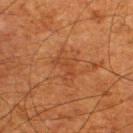Case summary:
* workup · total-body-photography surveillance lesion; no biopsy
* automated metrics · an area of roughly 5.5 mm², an outline eccentricity of about 0.75 (0 = round, 1 = elongated), and two-axis asymmetry of about 0.5; a mean CIELAB color near L≈37 a*≈23 b*≈33 and a lesion-to-skin contrast of about 4.5 (normalized; higher = more distinct); border irregularity of about 5.5 on a 0–10 scale, a within-lesion color-variation index near 1.5/10, and peripheral color asymmetry of about 0.5; a lesion-detection confidence of about 100/100
* site · the upper back
* patient · male, aged around 65
* acquisition · total-body-photography crop, ~15 mm field of view